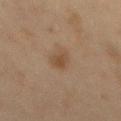{
  "biopsy_status": "not biopsied; imaged during a skin examination",
  "lesion_size": {
    "long_diameter_mm_approx": 3.0
  },
  "site": "back",
  "image": {
    "source": "total-body photography crop",
    "field_of_view_mm": 15
  },
  "patient": {
    "sex": "male",
    "age_approx": 45
  },
  "automated_metrics": {
    "area_mm2_approx": 5.0,
    "shape_asymmetry": 0.25,
    "cielab_L": 40,
    "cielab_a": 14,
    "cielab_b": 26,
    "vs_skin_darker_L": 7.0,
    "border_irregularity_0_10": 2.5,
    "color_variation_0_10": 1.5,
    "peripheral_color_asymmetry": 0.5
  },
  "lighting": "cross-polarized"
}